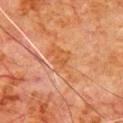The lesion was tiled from a total-body skin photograph and was not biopsied.